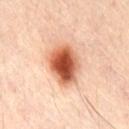Q: How large is the lesion?
A: about 5 mm
Q: How was this image acquired?
A: ~15 mm tile from a whole-body skin photo
Q: Automated lesion metrics?
A: a lesion area of about 15 mm², an outline eccentricity of about 0.65 (0 = round, 1 = elongated), and two-axis asymmetry of about 0.2; a lesion color around L≈57 a*≈28 b*≈35 in CIELAB, roughly 20 lightness units darker than nearby skin, and a normalized lesion–skin contrast near 12.5; internal color variation of about 8.5 on a 0–10 scale and a peripheral color-asymmetry measure near 2.5; a nevus-likeness score of about 100/100 and a lesion-detection confidence of about 100/100
Q: What is the anatomic site?
A: the chest
Q: Patient demographics?
A: male, aged around 35